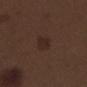{
  "biopsy_status": "not biopsied; imaged during a skin examination",
  "lighting": "white-light",
  "patient": {
    "sex": "male",
    "age_approx": 70
  },
  "lesion_size": {
    "long_diameter_mm_approx": 3.0
  },
  "automated_metrics": {
    "cielab_L": 24,
    "cielab_a": 15,
    "cielab_b": 21,
    "vs_skin_darker_L": 5.0,
    "vs_skin_contrast_norm": 6.0,
    "border_irregularity_0_10": 2.5,
    "color_variation_0_10": 1.5,
    "peripheral_color_asymmetry": 0.5,
    "lesion_detection_confidence_0_100": 100
  },
  "image": {
    "source": "total-body photography crop",
    "field_of_view_mm": 15
  },
  "site": "left thigh"
}